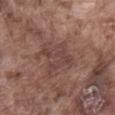workup: no biopsy performed (imaged during a skin exam); location: the front of the torso; acquisition: ~15 mm crop, total-body skin-cancer survey; diameter: about 4.5 mm; subject: male, in their mid-70s.